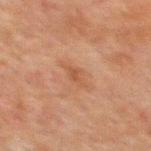{
  "biopsy_status": "not biopsied; imaged during a skin examination",
  "site": "mid back",
  "patient": {
    "sex": "male",
    "age_approx": 60
  },
  "image": {
    "source": "total-body photography crop",
    "field_of_view_mm": 15
  },
  "lighting": "cross-polarized"
}